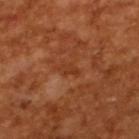Impression: Imaged during a routine full-body skin examination; the lesion was not biopsied and no histopathology is available. Image and clinical context: The total-body-photography lesion software estimated a detector confidence of about 100 out of 100 that the crop contains a lesion. A male subject aged around 65. Captured under cross-polarized illumination. About 2.5 mm across. A 15 mm close-up tile from a total-body photography series done for melanoma screening.